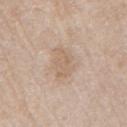This lesion was catalogued during total-body skin photography and was not selected for biopsy. The patient is a male aged approximately 60. A 15 mm crop from a total-body photograph taken for skin-cancer surveillance. The recorded lesion diameter is about 4 mm. The lesion is located on the right upper arm. Imaged with white-light lighting.